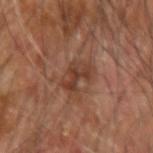Imaged during a routine full-body skin examination; the lesion was not biopsied and no histopathology is available. Longest diameter approximately 4 mm. From the right forearm. A male subject about 65 years old. Cropped from a total-body skin-imaging series; the visible field is about 15 mm. Imaged with cross-polarized lighting.